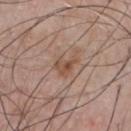<tbp_lesion>
  <biopsy_status>not biopsied; imaged during a skin examination</biopsy_status>
  <patient>
    <sex>male</sex>
    <age_approx>55</age_approx>
  </patient>
  <automated_metrics>
    <area_mm2_approx>5.0</area_mm2_approx>
    <eccentricity>0.65</eccentricity>
    <shape_asymmetry>0.45</shape_asymmetry>
  </automated_metrics>
  <site>chest</site>
  <lighting>white-light</lighting>
  <image>
    <source>total-body photography crop</source>
    <field_of_view_mm>15</field_of_view_mm>
  </image>
  <lesion_size>
    <long_diameter_mm_approx>3.0</long_diameter_mm_approx>
  </lesion_size>
</tbp_lesion>